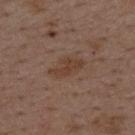notes: total-body-photography surveillance lesion; no biopsy | subject: male, aged 48 to 52 | site: the mid back | image: total-body-photography crop, ~15 mm field of view.